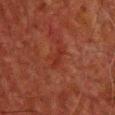Notes:
• size: ≈3 mm
• patient: male, about 60 years old
• location: the front of the torso
• image-analysis metrics: border irregularity of about 4 on a 0–10 scale and a within-lesion color-variation index near 0/10; lesion-presence confidence of about 95/100
• acquisition: 15 mm crop, total-body photography